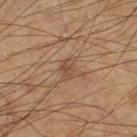Part of a total-body skin-imaging series; this lesion was reviewed on a skin check and was not flagged for biopsy. Cropped from a whole-body photographic skin survey; the tile spans about 15 mm. The tile uses cross-polarized illumination. The subject is a male about 70 years old. Measured at roughly 3 mm in maximum diameter. The lesion is located on the right thigh. The total-body-photography lesion software estimated a lesion color around L≈47 a*≈17 b*≈30 in CIELAB, roughly 7 lightness units darker than nearby skin, and a normalized lesion–skin contrast near 6. The analysis additionally found border irregularity of about 2.5 on a 0–10 scale and a within-lesion color-variation index near 2.5/10. The software also gave an automated nevus-likeness rating near 20 out of 100 and a lesion-detection confidence of about 100/100.